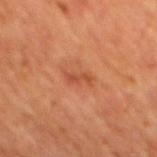{
  "biopsy_status": "not biopsied; imaged during a skin examination",
  "patient": {
    "sex": "male",
    "age_approx": 65
  },
  "site": "mid back",
  "automated_metrics": {
    "cielab_L": 45,
    "cielab_a": 28,
    "cielab_b": 34,
    "nevus_likeness_0_100": 5,
    "lesion_detection_confidence_0_100": 100
  },
  "lighting": "cross-polarized",
  "lesion_size": {
    "long_diameter_mm_approx": 3.0
  },
  "image": {
    "source": "total-body photography crop",
    "field_of_view_mm": 15
  }
}